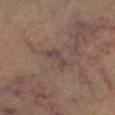{"biopsy_status": "not biopsied; imaged during a skin examination", "lighting": "cross-polarized", "automated_metrics": {"area_mm2_approx": 3.5, "eccentricity": 0.9, "shape_asymmetry": 0.4, "border_irregularity_0_10": 5.0, "color_variation_0_10": 1.0, "peripheral_color_asymmetry": 0.5, "lesion_detection_confidence_0_100": 95}, "site": "leg", "patient": {"sex": "male", "age_approx": 60}, "image": {"source": "total-body photography crop", "field_of_view_mm": 15}, "lesion_size": {"long_diameter_mm_approx": 3.0}}